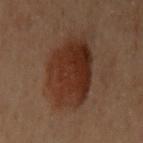Recorded during total-body skin imaging; not selected for excision or biopsy.
Captured under cross-polarized illumination.
Cropped from a total-body skin-imaging series; the visible field is about 15 mm.
The lesion is on the arm.
A female subject, approximately 60 years of age.
The lesion's longest dimension is about 7 mm.
An algorithmic analysis of the crop reported an area of roughly 31 mm², a shape eccentricity near 0.65, and a shape-asymmetry score of about 0.15 (0 = symmetric). The software also gave a border-irregularity index near 2/10, a within-lesion color-variation index near 5.5/10, and a peripheral color-asymmetry measure near 2. And it measured a classifier nevus-likeness of about 95/100 and a lesion-detection confidence of about 100/100.